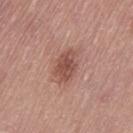Clinical impression:
Part of a total-body skin-imaging series; this lesion was reviewed on a skin check and was not flagged for biopsy.
Image and clinical context:
A female patient, aged 63–67. About 4 mm across. The lesion-visualizer software estimated an area of roughly 7.5 mm², a shape eccentricity near 0.8, and a symmetry-axis asymmetry near 0.2. It also reported an average lesion color of about L≈50 a*≈24 b*≈25 (CIELAB) and about 11 CIELAB-L* units darker than the surrounding skin. And it measured a border-irregularity rating of about 2.5/10, a within-lesion color-variation index near 3/10, and a peripheral color-asymmetry measure near 1. It also reported a detector confidence of about 100 out of 100 that the crop contains a lesion. On the left thigh. The tile uses white-light illumination. A close-up tile cropped from a whole-body skin photograph, about 15 mm across.